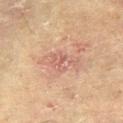<lesion>
  <biopsy_status>not biopsied; imaged during a skin examination</biopsy_status>
  <patient>
    <sex>male</sex>
    <age_approx>70</age_approx>
  </patient>
  <lighting>cross-polarized</lighting>
  <image>
    <source>total-body photography crop</source>
    <field_of_view_mm>15</field_of_view_mm>
  </image>
  <lesion_size>
    <long_diameter_mm_approx>3.5</long_diameter_mm_approx>
  </lesion_size>
  <site>right thigh</site>
</lesion>